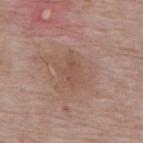Part of a total-body skin-imaging series; this lesion was reviewed on a skin check and was not flagged for biopsy. The subject is a male in their mid- to late 60s. The lesion is on the mid back. A 15 mm crop from a total-body photograph taken for skin-cancer surveillance. This is a white-light tile. The total-body-photography lesion software estimated a lesion-detection confidence of about 100/100. The recorded lesion diameter is about 4 mm.